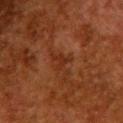About 2.5 mm across. The total-body-photography lesion software estimated a lesion color around L≈25 a*≈22 b*≈28 in CIELAB and a normalized lesion–skin contrast near 6. The analysis additionally found a classifier nevus-likeness of about 0/100 and a lesion-detection confidence of about 100/100. A roughly 15 mm field-of-view crop from a total-body skin photograph. The lesion is on the upper back. The tile uses cross-polarized illumination. The patient is a female aged around 50.